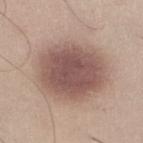No biopsy was performed on this lesion — it was imaged during a full skin examination and was not determined to be concerning. Located on the right lower leg. A 15 mm crop from a total-body photograph taken for skin-cancer surveillance. A male subject, about 45 years old. Approximately 7.5 mm at its widest.